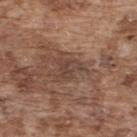Captured during whole-body skin photography for melanoma surveillance; the lesion was not biopsied. Captured under white-light illumination. A region of skin cropped from a whole-body photographic capture, roughly 15 mm wide. On the upper back. The subject is a male aged around 75.